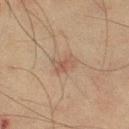Context:
A female subject, roughly 55 years of age. The lesion's longest dimension is about 3 mm. On the leg. Cropped from a total-body skin-imaging series; the visible field is about 15 mm. The lesion-visualizer software estimated an area of roughly 5 mm² and a symmetry-axis asymmetry near 0.25.